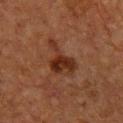Assessment: The lesion was photographed on a routine skin check and not biopsied; there is no pathology result. Clinical summary: The lesion is on the chest. The tile uses cross-polarized illumination. A male patient about 50 years old. A roughly 15 mm field-of-view crop from a total-body skin photograph. About 5 mm across. An algorithmic analysis of the crop reported a lesion area of about 9 mm² and an eccentricity of roughly 0.75. And it measured roughly 8 lightness units darker than nearby skin and a lesion-to-skin contrast of about 9 (normalized; higher = more distinct).